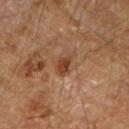{
  "biopsy_status": "not biopsied; imaged during a skin examination",
  "patient": {
    "sex": "male",
    "age_approx": "85+"
  },
  "image": {
    "source": "total-body photography crop",
    "field_of_view_mm": 15
  },
  "lesion_size": {
    "long_diameter_mm_approx": 3.0
  },
  "site": "arm"
}